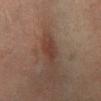The lesion was photographed on a routine skin check and not biopsied; there is no pathology result. A region of skin cropped from a whole-body photographic capture, roughly 15 mm wide. From the left lower leg. Longest diameter approximately 2.5 mm. This is a cross-polarized tile. A female subject, in their mid-50s.